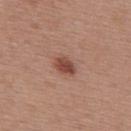notes: imaged on a skin check; not biopsied | illumination: white-light illumination | size: ≈3 mm | location: the upper back | image source: 15 mm crop, total-body photography | subject: female, aged 48–52.